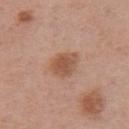biopsy status: imaged on a skin check; not biopsied
TBP lesion metrics: an area of roughly 7.5 mm² and a shape-asymmetry score of about 0.2 (0 = symmetric); an average lesion color of about L≈53 a*≈22 b*≈30 (CIELAB) and about 10 CIELAB-L* units darker than the surrounding skin; a color-variation rating of about 3/10 and peripheral color asymmetry of about 1; a nevus-likeness score of about 95/100
subject: male, roughly 70 years of age
image: total-body-photography crop, ~15 mm field of view
size: about 3 mm
tile lighting: white-light illumination
site: the abdomen